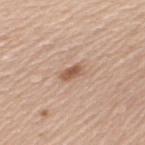Notes:
- workup · catalogued during a skin exam; not biopsied
- subject · female, aged approximately 60
- diameter · about 3 mm
- anatomic site · the upper back
- image-analysis metrics · an average lesion color of about L≈56 a*≈20 b*≈30 (CIELAB), about 12 CIELAB-L* units darker than the surrounding skin, and a normalized lesion–skin contrast near 8; a border-irregularity index near 2/10
- acquisition · 15 mm crop, total-body photography
- illumination · white-light illumination